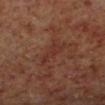This lesion was catalogued during total-body skin photography and was not selected for biopsy.
Located on the left lower leg.
This is a cross-polarized tile.
The total-body-photography lesion software estimated a footprint of about 5.5 mm² and a symmetry-axis asymmetry near 0.35.
A region of skin cropped from a whole-body photographic capture, roughly 15 mm wide.
A male subject aged 58–62.